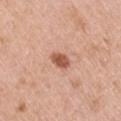The lesion was tiled from a total-body skin photograph and was not biopsied.
The lesion's longest dimension is about 3 mm.
Located on the left upper arm.
The tile uses white-light illumination.
This image is a 15 mm lesion crop taken from a total-body photograph.
A male subject approximately 40 years of age.